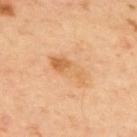{
  "biopsy_status": "not biopsied; imaged during a skin examination",
  "patient": {
    "sex": "male",
    "age_approx": 60
  },
  "image": {
    "source": "total-body photography crop",
    "field_of_view_mm": 15
  },
  "site": "upper back"
}